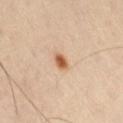Located on the leg. The patient is a male aged around 65. The tile uses cross-polarized illumination. Automated tile analysis of the lesion measured an area of roughly 3 mm², a shape eccentricity near 0.65, and a symmetry-axis asymmetry near 0.25. And it measured a border-irregularity index near 2/10, internal color variation of about 2.5 on a 0–10 scale, and peripheral color asymmetry of about 1. The recorded lesion diameter is about 2 mm. A 15 mm close-up tile from a total-body photography series done for melanoma screening.